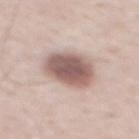Imaged during a routine full-body skin examination; the lesion was not biopsied and no histopathology is available.